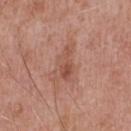Captured during whole-body skin photography for melanoma surveillance; the lesion was not biopsied.
The total-body-photography lesion software estimated a nevus-likeness score of about 0/100 and a detector confidence of about 100 out of 100 that the crop contains a lesion.
The lesion is located on the chest.
A lesion tile, about 15 mm wide, cut from a 3D total-body photograph.
A male patient in their 70s.